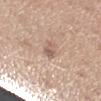Captured during whole-body skin photography for melanoma surveillance; the lesion was not biopsied. A lesion tile, about 15 mm wide, cut from a 3D total-body photograph. The lesion is on the left forearm. The tile uses white-light illumination. The patient is a female approximately 40 years of age. An algorithmic analysis of the crop reported a nevus-likeness score of about 5/100. Approximately 2.5 mm at its widest.